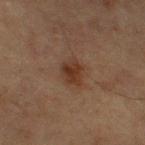workup: no biopsy performed (imaged during a skin exam)
body site: the left thigh
lesion size: ~3.5 mm (longest diameter)
image: ~15 mm tile from a whole-body skin photo
tile lighting: cross-polarized illumination
subject: male, about 75 years old